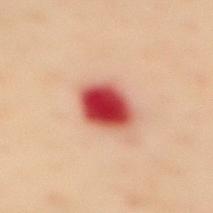The lesion was tiled from a total-body skin photograph and was not biopsied. The lesion's longest dimension is about 4 mm. A female patient, roughly 60 years of age. The lesion is on the mid back. This image is a 15 mm lesion crop taken from a total-body photograph.